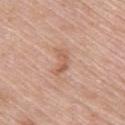follow-up = catalogued during a skin exam; not biopsied
patient = female, in their mid- to late 60s
acquisition = 15 mm crop, total-body photography
location = the upper back
illumination = white-light
TBP lesion metrics = a mean CIELAB color near L≈59 a*≈22 b*≈31; a border-irregularity rating of about 5/10